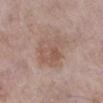Findings:
* biopsy status: catalogued during a skin exam; not biopsied
* body site: the leg
* image: total-body-photography crop, ~15 mm field of view
* illumination: white-light
* subject: female, aged approximately 70
* size: ~5 mm (longest diameter)